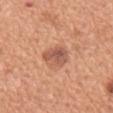Impression: Captured during whole-body skin photography for melanoma surveillance; the lesion was not biopsied. Context: The tile uses white-light illumination. A 15 mm crop from a total-body photograph taken for skin-cancer surveillance. Approximately 3 mm at its widest. The patient is a male aged around 65. The total-body-photography lesion software estimated a lesion area of about 7.5 mm², an outline eccentricity of about 0.25 (0 = round, 1 = elongated), and a symmetry-axis asymmetry near 0.2. It also reported a lesion–skin lightness drop of about 11 and a normalized lesion–skin contrast near 7. And it measured a border-irregularity index near 2/10, a within-lesion color-variation index near 5.5/10, and radial color variation of about 2. On the abdomen.